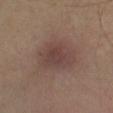Recorded during total-body skin imaging; not selected for excision or biopsy. A region of skin cropped from a whole-body photographic capture, roughly 15 mm wide. Measured at roughly 5 mm in maximum diameter. A patient aged 53–57. On the right lower leg. Automated tile analysis of the lesion measured an eccentricity of roughly 0.75 and two-axis asymmetry of about 0.2. And it measured a border-irregularity rating of about 2/10, a color-variation rating of about 2.5/10, and radial color variation of about 1. And it measured a detector confidence of about 100 out of 100 that the crop contains a lesion.